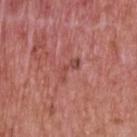A male subject in their 60s.
A region of skin cropped from a whole-body photographic capture, roughly 15 mm wide.
Captured under white-light illumination.
About 3.5 mm across.
An algorithmic analysis of the crop reported a footprint of about 3.5 mm², a shape eccentricity near 0.95, and two-axis asymmetry of about 0.6. It also reported a border-irregularity index near 8/10, a color-variation rating of about 0/10, and a peripheral color-asymmetry measure near 0.
The lesion is on the upper back.